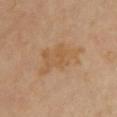Clinical impression:
Captured during whole-body skin photography for melanoma surveillance; the lesion was not biopsied.
Clinical summary:
Captured under cross-polarized illumination. The subject is a female aged 43 to 47. On the chest. Measured at roughly 6.5 mm in maximum diameter. A region of skin cropped from a whole-body photographic capture, roughly 15 mm wide.